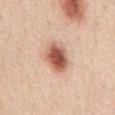Imaged during a routine full-body skin examination; the lesion was not biopsied and no histopathology is available. A roughly 15 mm field-of-view crop from a total-body skin photograph. A female patient roughly 45 years of age. Automated image analysis of the tile measured a footprint of about 11 mm², a shape eccentricity near 0.65, and two-axis asymmetry of about 0.15. The software also gave a lesion-to-skin contrast of about 10.5 (normalized; higher = more distinct). It also reported internal color variation of about 7.5 on a 0–10 scale and radial color variation of about 2. This is a white-light tile. The lesion is on the chest. Longest diameter approximately 4 mm.